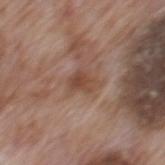Longest diameter approximately 3.5 mm.
The lesion is located on the mid back.
A male subject, aged approximately 70.
Captured under white-light illumination.
A roughly 15 mm field-of-view crop from a total-body skin photograph.